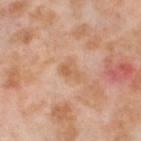{
  "biopsy_status": "not biopsied; imaged during a skin examination",
  "patient": {
    "sex": "female",
    "age_approx": 55
  },
  "lighting": "cross-polarized",
  "site": "left thigh",
  "automated_metrics": {
    "vs_skin_darker_L": 8.0,
    "vs_skin_contrast_norm": 6.0,
    "peripheral_color_asymmetry": 0.0,
    "nevus_likeness_0_100": 0
  },
  "image": {
    "source": "total-body photography crop",
    "field_of_view_mm": 15
  },
  "lesion_size": {
    "long_diameter_mm_approx": 2.5
  }
}